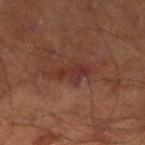Recorded during total-body skin imaging; not selected for excision or biopsy.
This image is a 15 mm lesion crop taken from a total-body photograph.
Approximately 4.5 mm at its widest.
The lesion-visualizer software estimated a lesion area of about 6 mm², a shape eccentricity near 0.9, and two-axis asymmetry of about 0.4. The software also gave an average lesion color of about L≈26 a*≈20 b*≈20 (CIELAB), a lesion–skin lightness drop of about 6, and a normalized lesion–skin contrast near 6.5.
Located on the left lower leg.
This is a cross-polarized tile.
A male patient, about 70 years old.